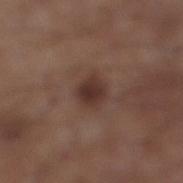Part of a total-body skin-imaging series; this lesion was reviewed on a skin check and was not flagged for biopsy. Longest diameter approximately 2.5 mm. On the right lower leg. A region of skin cropped from a whole-body photographic capture, roughly 15 mm wide. A male patient, in their mid- to late 50s. Captured under white-light illumination. The lesion-visualizer software estimated a lesion area of about 5.5 mm², an outline eccentricity of about 0.5 (0 = round, 1 = elongated), and a shape-asymmetry score of about 0.15 (0 = symmetric). The software also gave a mean CIELAB color near L≈33 a*≈18 b*≈22, a lesion–skin lightness drop of about 10, and a normalized lesion–skin contrast near 9. The software also gave a border-irregularity index near 1.5/10, a within-lesion color-variation index near 3/10, and a peripheral color-asymmetry measure near 1. The analysis additionally found a detector confidence of about 100 out of 100 that the crop contains a lesion.